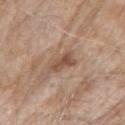  biopsy_status: not biopsied; imaged during a skin examination
  patient:
    sex: female
    age_approx: 85+
  site: right upper arm
  lighting: white-light
  lesion_size:
    long_diameter_mm_approx: 3.5
  image:
    source: total-body photography crop
    field_of_view_mm: 15